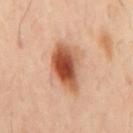<record>
<site>mid back</site>
<patient>
  <sex>male</sex>
  <age_approx>40</age_approx>
</patient>
<image>
  <source>total-body photography crop</source>
  <field_of_view_mm>15</field_of_view_mm>
</image>
</record>